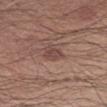Recorded during total-body skin imaging; not selected for excision or biopsy. The lesion-visualizer software estimated an automated nevus-likeness rating near 5 out of 100 and a lesion-detection confidence of about 100/100. The lesion is on the left forearm. A male patient aged approximately 30. Cropped from a total-body skin-imaging series; the visible field is about 15 mm. Approximately 2.5 mm at its widest.